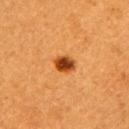No biopsy was performed on this lesion — it was imaged during a full skin examination and was not determined to be concerning.
A female subject about 55 years old.
This is a cross-polarized tile.
On the arm.
Automated image analysis of the tile measured an area of roughly 4.5 mm², a shape eccentricity near 0.65, and a shape-asymmetry score of about 0.25 (0 = symmetric). The software also gave a border-irregularity rating of about 2/10, a within-lesion color-variation index near 3.5/10, and a peripheral color-asymmetry measure near 1.
Approximately 2.5 mm at its widest.
This image is a 15 mm lesion crop taken from a total-body photograph.